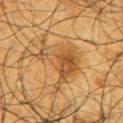{
  "biopsy_status": "not biopsied; imaged during a skin examination",
  "patient": {
    "sex": "male",
    "age_approx": 65
  },
  "site": "arm",
  "lesion_size": {
    "long_diameter_mm_approx": 6.0
  },
  "automated_metrics": {
    "nevus_likeness_0_100": 90
  },
  "lighting": "cross-polarized",
  "image": {
    "source": "total-body photography crop",
    "field_of_view_mm": 15
  }
}